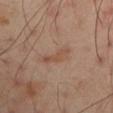Part of a total-body skin-imaging series; this lesion was reviewed on a skin check and was not flagged for biopsy. The lesion is located on the left arm. A 15 mm close-up extracted from a 3D total-body photography capture. The subject is a male roughly 50 years of age.